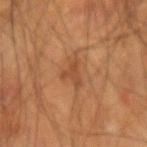Imaged during a routine full-body skin examination; the lesion was not biopsied and no histopathology is available.
A male subject in their mid-60s.
A lesion tile, about 15 mm wide, cut from a 3D total-body photograph.
Located on the left forearm.
The total-body-photography lesion software estimated an area of roughly 3.5 mm², a shape eccentricity near 0.6, and a symmetry-axis asymmetry near 0.65. The analysis additionally found a lesion–skin lightness drop of about 7 and a lesion-to-skin contrast of about 6 (normalized; higher = more distinct).
The tile uses cross-polarized illumination.
About 3 mm across.